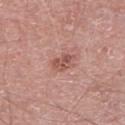This lesion was catalogued during total-body skin photography and was not selected for biopsy. Cropped from a whole-body photographic skin survey; the tile spans about 15 mm. An algorithmic analysis of the crop reported a mean CIELAB color near L≈53 a*≈24 b*≈26 and a lesion–skin lightness drop of about 10. It also reported an automated nevus-likeness rating near 15 out of 100 and a detector confidence of about 100 out of 100 that the crop contains a lesion. A male subject approximately 65 years of age. Approximately 3 mm at its widest. The lesion is on the right lower leg.